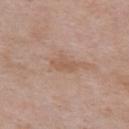Clinical impression: Recorded during total-body skin imaging; not selected for excision or biopsy. Image and clinical context: From the chest. This is a white-light tile. Approximately 3 mm at its widest. A male patient, aged around 55. A 15 mm close-up tile from a total-body photography series done for melanoma screening. An algorithmic analysis of the crop reported a lesion color around L≈56 a*≈19 b*≈30 in CIELAB. The analysis additionally found a border-irregularity rating of about 4/10, internal color variation of about 1 on a 0–10 scale, and peripheral color asymmetry of about 0.5.